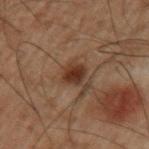A lesion tile, about 15 mm wide, cut from a 3D total-body photograph.
The lesion's longest dimension is about 3 mm.
On the left upper arm.
The subject is a male aged 48 to 52.
This is a cross-polarized tile.